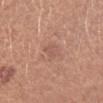notes = imaged on a skin check; not biopsied
tile lighting = white-light illumination
image source = ~15 mm crop, total-body skin-cancer survey
subject = female, aged approximately 25
location = the left forearm
lesion diameter = ≈2.5 mm